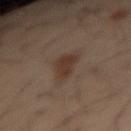A 15 mm close-up tile from a total-body photography series done for melanoma screening.
The total-body-photography lesion software estimated a lesion area of about 7 mm², a shape eccentricity near 0.85, and a symmetry-axis asymmetry near 0.35. The software also gave a border-irregularity index near 3.5/10, internal color variation of about 2 on a 0–10 scale, and a peripheral color-asymmetry measure near 0.5.
Imaged with cross-polarized lighting.
A male subject aged around 40.
The lesion's longest dimension is about 4 mm.
Located on the right forearm.